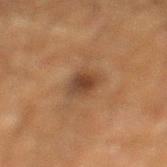No biopsy was performed on this lesion — it was imaged during a full skin examination and was not determined to be concerning. A male subject approximately 65 years of age. The lesion is located on the left lower leg. A region of skin cropped from a whole-body photographic capture, roughly 15 mm wide. About 2.5 mm across. Automated image analysis of the tile measured an average lesion color of about L≈35 a*≈17 b*≈27 (CIELAB), a lesion–skin lightness drop of about 9, and a lesion-to-skin contrast of about 8.5 (normalized; higher = more distinct). It also reported a color-variation rating of about 4/10 and radial color variation of about 1.5.